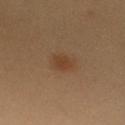biopsy_status: not biopsied; imaged during a skin examination
automated_metrics:
  cielab_L: 40
  cielab_a: 18
  cielab_b: 31
  vs_skin_darker_L: 6.0
  vs_skin_contrast_norm: 6.0
  border_irregularity_0_10: 2.0
  color_variation_0_10: 1.5
  peripheral_color_asymmetry: 0.5
patient:
  sex: female
  age_approx: 50
image:
  source: total-body photography crop
  field_of_view_mm: 15
site: right upper arm
lighting: cross-polarized
lesion_size:
  long_diameter_mm_approx: 3.0